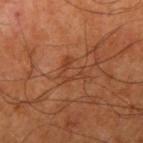Impression: The lesion was photographed on a routine skin check and not biopsied; there is no pathology result. Context: The recorded lesion diameter is about 3 mm. On the right upper arm. A lesion tile, about 15 mm wide, cut from a 3D total-body photograph. Imaged with cross-polarized lighting. The patient is a male aged approximately 65. An algorithmic analysis of the crop reported a border-irregularity index near 7/10, a color-variation rating of about 2/10, and a peripheral color-asymmetry measure near 0.5. And it measured a nevus-likeness score of about 0/100 and lesion-presence confidence of about 100/100.